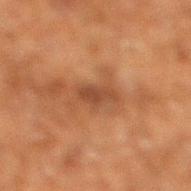Recorded during total-body skin imaging; not selected for excision or biopsy.
Automated tile analysis of the lesion measured an outline eccentricity of about 0.9 (0 = round, 1 = elongated) and a symmetry-axis asymmetry near 0.25.
From the right lower leg.
Approximately 3.5 mm at its widest.
This image is a 15 mm lesion crop taken from a total-body photograph.
A male subject, aged 58–62.